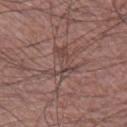Case summary:
• biopsy status · catalogued during a skin exam; not biopsied
• patient · male, roughly 60 years of age
• acquisition · ~15 mm crop, total-body skin-cancer survey
• illumination · white-light illumination
• location · the leg
• diameter · about 4 mm